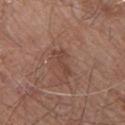- anatomic site: the upper back
- acquisition: 15 mm crop, total-body photography
- patient: male, in their mid-50s